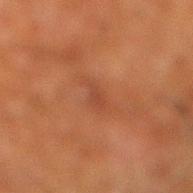follow-up: no biopsy performed (imaged during a skin exam)
lighting: cross-polarized
image: 15 mm crop, total-body photography
subject: male, aged 63 to 67
site: the right lower leg
size: ≈3 mm